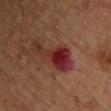workup: no biopsy performed (imaged during a skin exam) | subject: female, roughly 70 years of age | image: 15 mm crop, total-body photography | lesion size: ≈6.5 mm | body site: the upper back | tile lighting: cross-polarized | automated metrics: a border-irregularity rating of about 8.5/10, a color-variation rating of about 5/10, and a peripheral color-asymmetry measure near 1; an automated nevus-likeness rating near 0 out of 100 and a detector confidence of about 100 out of 100 that the crop contains a lesion.